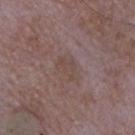Part of a total-body skin-imaging series; this lesion was reviewed on a skin check and was not flagged for biopsy. A region of skin cropped from a whole-body photographic capture, roughly 15 mm wide. An algorithmic analysis of the crop reported an area of roughly 5 mm², an eccentricity of roughly 0.8, and a shape-asymmetry score of about 0.35 (0 = symmetric). Measured at roughly 3.5 mm in maximum diameter. From the chest. The tile uses white-light illumination. The patient is a male aged 63 to 67.